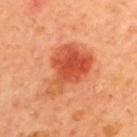Q: Was a biopsy performed?
A: catalogued during a skin exam; not biopsied
Q: What kind of image is this?
A: ~15 mm tile from a whole-body skin photo
Q: Who is the patient?
A: male, in their mid-60s
Q: Lesion location?
A: the upper back